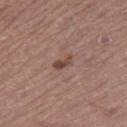Impression: Captured during whole-body skin photography for melanoma surveillance; the lesion was not biopsied. Acquisition and patient details: The tile uses white-light illumination. A male subject, aged around 65. A lesion tile, about 15 mm wide, cut from a 3D total-body photograph. Longest diameter approximately 2.5 mm. From the right thigh.